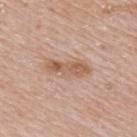notes: catalogued during a skin exam; not biopsied
patient: male, aged around 75
lesion diameter: ~5 mm (longest diameter)
site: the back
image-analysis metrics: about 10 CIELAB-L* units darker than the surrounding skin and a normalized border contrast of about 7; a border-irregularity rating of about 4/10
acquisition: total-body-photography crop, ~15 mm field of view